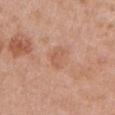Impression: This lesion was catalogued during total-body skin photography and was not selected for biopsy. Background: The lesion is located on the left upper arm. A 15 mm crop from a total-body photograph taken for skin-cancer surveillance. The lesion's longest dimension is about 3 mm. Captured under white-light illumination. The subject is a female aged 48–52. The total-body-photography lesion software estimated a lesion color around L≈58 a*≈24 b*≈32 in CIELAB, about 7 CIELAB-L* units darker than the surrounding skin, and a lesion-to-skin contrast of about 5 (normalized; higher = more distinct). It also reported a detector confidence of about 100 out of 100 that the crop contains a lesion.